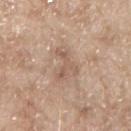Recorded during total-body skin imaging; not selected for excision or biopsy. A male patient aged 63–67. A 15 mm crop from a total-body photograph taken for skin-cancer surveillance. The lesion is located on the left upper arm. Longest diameter approximately 4 mm.